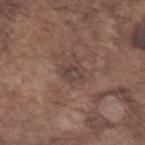Imaged during a routine full-body skin examination; the lesion was not biopsied and no histopathology is available. Imaged with white-light lighting. The subject is a male approximately 75 years of age. About 2.5 mm across. A 15 mm close-up tile from a total-body photography series done for melanoma screening. The lesion is located on the left forearm.